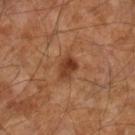A close-up tile cropped from a whole-body skin photograph, about 15 mm across. The subject is a male aged approximately 60. On the right lower leg.